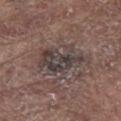Findings:
* notes: catalogued during a skin exam; not biopsied
* lesion size: ≈6.5 mm
* illumination: white-light
* location: the chest
* patient: male, aged 78–82
* image: total-body-photography crop, ~15 mm field of view
* automated metrics: an outline eccentricity of about 0.85 (0 = round, 1 = elongated) and a symmetry-axis asymmetry near 0.35